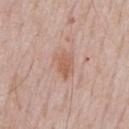biopsy status: no biopsy performed (imaged during a skin exam)
patient: male, approximately 60 years of age
site: the chest
image source: 15 mm crop, total-body photography
illumination: white-light
lesion size: ~3.5 mm (longest diameter)